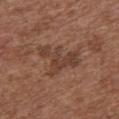Imaged during a routine full-body skin examination; the lesion was not biopsied and no histopathology is available.
The subject is a female aged around 75.
The tile uses white-light illumination.
About 5 mm across.
The lesion is on the chest.
A roughly 15 mm field-of-view crop from a total-body skin photograph.
Automated image analysis of the tile measured an area of roughly 12 mm², an eccentricity of roughly 0.65, and two-axis asymmetry of about 0.65. The software also gave a lesion–skin lightness drop of about 8 and a lesion-to-skin contrast of about 6.5 (normalized; higher = more distinct).